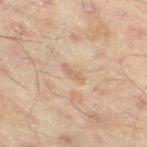{
  "biopsy_status": "not biopsied; imaged during a skin examination",
  "image": {
    "source": "total-body photography crop",
    "field_of_view_mm": 15
  },
  "site": "leg",
  "lighting": "cross-polarized",
  "patient": {
    "sex": "male",
    "age_approx": 45
  },
  "automated_metrics": {
    "area_mm2_approx": 2.5,
    "eccentricity": 0.95,
    "shape_asymmetry": 0.45,
    "border_irregularity_0_10": 4.5,
    "color_variation_0_10": 0.0,
    "peripheral_color_asymmetry": 0.0
  },
  "lesion_size": {
    "long_diameter_mm_approx": 2.5
  }
}